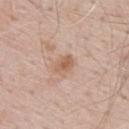Case summary:
* workup · no biopsy performed (imaged during a skin exam)